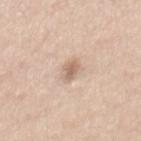This lesion was catalogued during total-body skin photography and was not selected for biopsy. On the lower back. A male patient, in their mid-30s. A lesion tile, about 15 mm wide, cut from a 3D total-body photograph. An algorithmic analysis of the crop reported a footprint of about 3.5 mm² and an eccentricity of roughly 0.8. The analysis additionally found a nevus-likeness score of about 35/100. Measured at roughly 2.5 mm in maximum diameter.